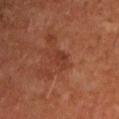subject = male, aged around 60 | site = the left upper arm | image = total-body-photography crop, ~15 mm field of view.